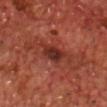Recorded during total-body skin imaging; not selected for excision or biopsy. The lesion is on the chest. A male subject, aged 68–72. The recorded lesion diameter is about 3.5 mm. This is a cross-polarized tile. A 15 mm crop from a total-body photograph taken for skin-cancer surveillance.